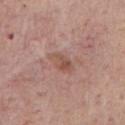• automated metrics — a shape eccentricity near 0.85 and two-axis asymmetry of about 0.25; a mean CIELAB color near L≈52 a*≈21 b*≈26, about 8 CIELAB-L* units darker than the surrounding skin, and a normalized lesion–skin contrast near 6.5; an automated nevus-likeness rating near 0 out of 100 and a lesion-detection confidence of about 100/100
• tile lighting — white-light illumination
• subject — male, in their mid- to late 70s
• imaging modality — total-body-photography crop, ~15 mm field of view
• location — the chest